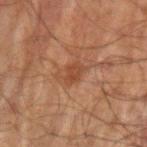workup = catalogued during a skin exam; not biopsied | acquisition = 15 mm crop, total-body photography | tile lighting = cross-polarized illumination | site = the left upper arm | subject = male, aged 68 to 72.